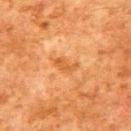notes: total-body-photography surveillance lesion; no biopsy
site: the upper back
tile lighting: cross-polarized illumination
lesion diameter: about 3.5 mm
imaging modality: ~15 mm crop, total-body skin-cancer survey
image-analysis metrics: a lesion area of about 4 mm², an eccentricity of roughly 0.85, and two-axis asymmetry of about 0.4; an average lesion color of about L≈46 a*≈23 b*≈38 (CIELAB) and a normalized border contrast of about 6; border irregularity of about 4.5 on a 0–10 scale and peripheral color asymmetry of about 0
patient: male, aged 78–82